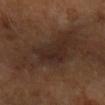workup: catalogued during a skin exam; not biopsied
lesion size: about 10 mm
location: the arm
patient: female
imaging modality: ~15 mm crop, total-body skin-cancer survey
lighting: cross-polarized
automated metrics: a lesion color around L≈28 a*≈16 b*≈23 in CIELAB and roughly 6 lightness units darker than nearby skin; a border-irregularity index near 5/10, a color-variation rating of about 4/10, and a peripheral color-asymmetry measure near 1